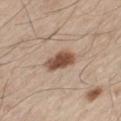This lesion was catalogued during total-body skin photography and was not selected for biopsy. The lesion is located on the left thigh. Automated image analysis of the tile measured a footprint of about 7.5 mm², a shape eccentricity near 0.7, and a symmetry-axis asymmetry near 0.2. The analysis additionally found border irregularity of about 2 on a 0–10 scale, internal color variation of about 4 on a 0–10 scale, and radial color variation of about 1. A region of skin cropped from a whole-body photographic capture, roughly 15 mm wide. This is a white-light tile. The lesion's longest dimension is about 3.5 mm. A male patient aged 58 to 62.